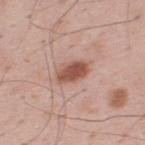Q: Is there a histopathology result?
A: total-body-photography surveillance lesion; no biopsy
Q: How large is the lesion?
A: about 3.5 mm
Q: What is the anatomic site?
A: the upper back
Q: Patient demographics?
A: male, in their mid-30s
Q: How was this image acquired?
A: ~15 mm crop, total-body skin-cancer survey